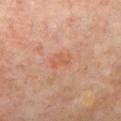follow-up=total-body-photography surveillance lesion; no biopsy
lesion size=~3 mm (longest diameter)
acquisition=~15 mm crop, total-body skin-cancer survey
patient=aged 63 to 67
lighting=cross-polarized illumination
anatomic site=the chest